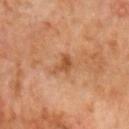- notes · catalogued during a skin exam; not biopsied
- acquisition · total-body-photography crop, ~15 mm field of view
- site · the head or neck
- lesion size · about 3 mm
- subject · male, roughly 60 years of age
- lighting · cross-polarized illumination